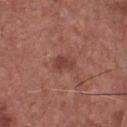Imaged during a routine full-body skin examination; the lesion was not biopsied and no histopathology is available. Approximately 2.5 mm at its widest. This is a white-light tile. A 15 mm close-up tile from a total-body photography series done for melanoma screening. On the chest. Automated image analysis of the tile measured a footprint of about 3.5 mm² and a symmetry-axis asymmetry near 0.4. And it measured a lesion–skin lightness drop of about 8 and a normalized border contrast of about 6.5. The software also gave a border-irregularity rating of about 3.5/10, internal color variation of about 1 on a 0–10 scale, and peripheral color asymmetry of about 0.5. It also reported a classifier nevus-likeness of about 5/100. A male subject aged around 75.